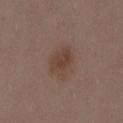The lesion was tiled from a total-body skin photograph and was not biopsied. Measured at roughly 3.5 mm in maximum diameter. Located on the mid back. This is a white-light tile. A female subject, aged around 30. A 15 mm crop from a total-body photograph taken for skin-cancer surveillance. An algorithmic analysis of the crop reported a lesion area of about 8 mm², an eccentricity of roughly 0.65, and two-axis asymmetry of about 0.2. The analysis additionally found a classifier nevus-likeness of about 80/100 and a lesion-detection confidence of about 100/100.